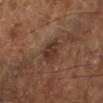Part of a total-body skin-imaging series; this lesion was reviewed on a skin check and was not flagged for biopsy. A lesion tile, about 15 mm wide, cut from a 3D total-body photograph. On the left lower leg. The tile uses cross-polarized illumination. Approximately 3 mm at its widest. The subject is about 65 years old.